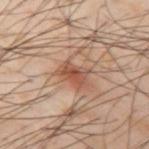Clinical impression: The lesion was tiled from a total-body skin photograph and was not biopsied. Image and clinical context: From the right upper arm. About 3.5 mm across. A lesion tile, about 15 mm wide, cut from a 3D total-body photograph. This is a cross-polarized tile. Automated tile analysis of the lesion measured a footprint of about 6.5 mm² and an outline eccentricity of about 0.75 (0 = round, 1 = elongated). The software also gave roughly 11 lightness units darker than nearby skin and a lesion-to-skin contrast of about 7.5 (normalized; higher = more distinct). The software also gave peripheral color asymmetry of about 1.5. The analysis additionally found a nevus-likeness score of about 85/100 and lesion-presence confidence of about 100/100. A male subject about 40 years old.